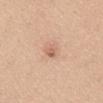No biopsy was performed on this lesion — it was imaged during a full skin examination and was not determined to be concerning. A female patient, approximately 40 years of age. The lesion is located on the mid back. About 2.5 mm across. A 15 mm close-up extracted from a 3D total-body photography capture. Automated image analysis of the tile measured an area of roughly 3 mm², an outline eccentricity of about 0.8 (0 = round, 1 = elongated), and a shape-asymmetry score of about 0.3 (0 = symmetric). The analysis additionally found a classifier nevus-likeness of about 10/100 and lesion-presence confidence of about 100/100.